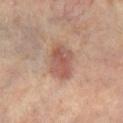  biopsy_status: not biopsied; imaged during a skin examination
  lesion_size:
    long_diameter_mm_approx: 4.5
  image:
    source: total-body photography crop
    field_of_view_mm: 15
  site: leg
  patient:
    sex: female
    age_approx: 65